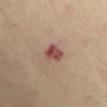biopsy status=no biopsy performed (imaged during a skin exam) | illumination=cross-polarized illumination | lesion size=~3 mm (longest diameter) | subject=female, roughly 40 years of age | TBP lesion metrics=a footprint of about 5 mm² and a symmetry-axis asymmetry near 0.25; a within-lesion color-variation index near 6/10 and peripheral color asymmetry of about 2 | imaging modality=total-body-photography crop, ~15 mm field of view | location=the chest.